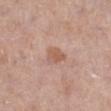Notes:
• follow-up — imaged on a skin check; not biopsied
• patient — female, aged around 55
• automated lesion analysis — an outline eccentricity of about 0.6 (0 = round, 1 = elongated) and a symmetry-axis asymmetry near 0.3
• size — ~2.5 mm (longest diameter)
• acquisition — ~15 mm crop, total-body skin-cancer survey
• location — the left lower leg
• illumination — white-light illumination